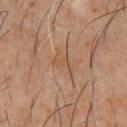Findings:
• workup · catalogued during a skin exam; not biopsied
• location · the front of the torso
• imaging modality · total-body-photography crop, ~15 mm field of view
• tile lighting · cross-polarized illumination
• patient · male, aged around 60
• lesion size · ≈3 mm